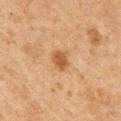{"biopsy_status": "not biopsied; imaged during a skin examination", "image": {"source": "total-body photography crop", "field_of_view_mm": 15}, "site": "chest", "patient": {"sex": "male", "age_approx": 75}}